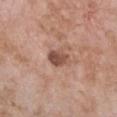Part of a total-body skin-imaging series; this lesion was reviewed on a skin check and was not flagged for biopsy.
Imaged with white-light lighting.
Located on the front of the torso.
The total-body-photography lesion software estimated a lesion area of about 5.5 mm² and a symmetry-axis asymmetry near 0.15. It also reported a mean CIELAB color near L≈50 a*≈21 b*≈27, roughly 13 lightness units darker than nearby skin, and a normalized lesion–skin contrast near 9. The software also gave a color-variation rating of about 4/10 and radial color variation of about 1.5. It also reported a lesion-detection confidence of about 100/100.
A roughly 15 mm field-of-view crop from a total-body skin photograph.
A female patient approximately 75 years of age.